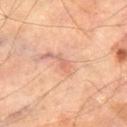Assessment: Captured during whole-body skin photography for melanoma surveillance; the lesion was not biopsied. Acquisition and patient details: A 15 mm close-up tile from a total-body photography series done for melanoma screening. Approximately 2.5 mm at its widest. The tile uses cross-polarized illumination. The lesion is located on the leg. A male subject aged 68 to 72.